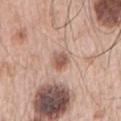Q: How was this image acquired?
A: ~15 mm crop, total-body skin-cancer survey
Q: Automated lesion metrics?
A: an area of roughly 4 mm², a shape eccentricity near 0.65, and two-axis asymmetry of about 0.2; internal color variation of about 3.5 on a 0–10 scale; a lesion-detection confidence of about 100/100
Q: How large is the lesion?
A: ≈2.5 mm
Q: Where on the body is the lesion?
A: the right upper arm
Q: Patient demographics?
A: male, approximately 65 years of age
Q: Illumination type?
A: white-light illumination